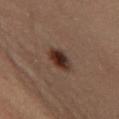The lesion was photographed on a routine skin check and not biopsied; there is no pathology result. Cropped from a whole-body photographic skin survey; the tile spans about 15 mm. This is a cross-polarized tile. The lesion is located on the left thigh. The patient is a female in their 40s.